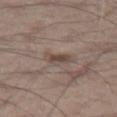| key | value |
|---|---|
| follow-up | total-body-photography surveillance lesion; no biopsy |
| site | the left thigh |
| patient | male, aged approximately 55 |
| image | ~15 mm tile from a whole-body skin photo |